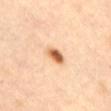{"biopsy_status": "not biopsied; imaged during a skin examination", "automated_metrics": {"eccentricity": 0.75, "shape_asymmetry": 0.2, "nevus_likeness_0_100": 100}, "image": {"source": "total-body photography crop", "field_of_view_mm": 15}, "site": "front of the torso", "lighting": "cross-polarized", "patient": {"sex": "female", "age_approx": 60}}